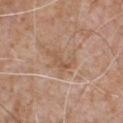biopsy_status: not biopsied; imaged during a skin examination
site: chest
image:
  source: total-body photography crop
  field_of_view_mm: 15
patient:
  sex: male
  age_approx: 65